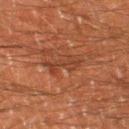<tbp_lesion>
<biopsy_status>not biopsied; imaged during a skin examination</biopsy_status>
<image>
  <source>total-body photography crop</source>
  <field_of_view_mm>15</field_of_view_mm>
</image>
<patient>
  <sex>male</sex>
  <age_approx>60</age_approx>
</patient>
<lighting>cross-polarized</lighting>
<site>left thigh</site>
<lesion_size>
  <long_diameter_mm_approx>4.5</long_diameter_mm_approx>
</lesion_size>
</tbp_lesion>